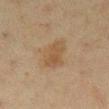Case summary:
* workup · imaged on a skin check; not biopsied
* subject · female, in their 50s
* location · the right lower leg
* acquisition · 15 mm crop, total-body photography